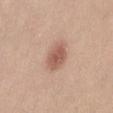<tbp_lesion>
<biopsy_status>not biopsied; imaged during a skin examination</biopsy_status>
<patient>
  <sex>female</sex>
  <age_approx>20</age_approx>
</patient>
<image>
  <source>total-body photography crop</source>
  <field_of_view_mm>15</field_of_view_mm>
</image>
<site>abdomen</site>
</tbp_lesion>